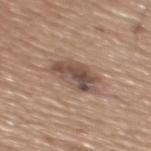Q: Was a biopsy performed?
A: no biopsy performed (imaged during a skin exam)